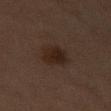Clinical impression: The lesion was tiled from a total-body skin photograph and was not biopsied. Background: The lesion is located on the left thigh. A female patient roughly 70 years of age. The lesion-visualizer software estimated a lesion area of about 7 mm², an eccentricity of roughly 0.5, and a shape-asymmetry score of about 0.15 (0 = symmetric). The software also gave about 6 CIELAB-L* units darker than the surrounding skin and a normalized lesion–skin contrast near 8.5. The analysis additionally found a color-variation rating of about 2.5/10 and radial color variation of about 1. The lesion's longest dimension is about 3.5 mm. Imaged with cross-polarized lighting. A roughly 15 mm field-of-view crop from a total-body skin photograph.